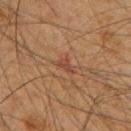| feature | finding |
|---|---|
| biopsy status | catalogued during a skin exam; not biopsied |
| site | the upper back |
| illumination | cross-polarized |
| lesion diameter | ~2.5 mm (longest diameter) |
| imaging modality | total-body-photography crop, ~15 mm field of view |
| subject | male, in their mid-60s |